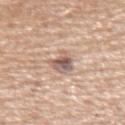No biopsy was performed on this lesion — it was imaged during a full skin examination and was not determined to be concerning.
Longest diameter approximately 3.5 mm.
From the right forearm.
A male patient in their mid- to late 50s.
A 15 mm close-up tile from a total-body photography series done for melanoma screening.
The tile uses white-light illumination.